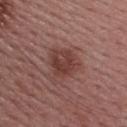  biopsy_status: not biopsied; imaged during a skin examination
  site: back
  lesion_size:
    long_diameter_mm_approx: 4.0
  lighting: white-light
  image:
    source: total-body photography crop
    field_of_view_mm: 15
  patient:
    sex: female
    age_approx: 55